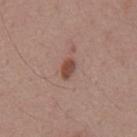{"biopsy_status": "not biopsied; imaged during a skin examination", "site": "mid back", "image": {"source": "total-body photography crop", "field_of_view_mm": 15}, "lighting": "white-light", "lesion_size": {"long_diameter_mm_approx": 2.5}, "automated_metrics": {"area_mm2_approx": 3.5, "eccentricity": 0.8, "border_irregularity_0_10": 1.5, "color_variation_0_10": 2.5, "peripheral_color_asymmetry": 1.0}, "patient": {"sex": "male", "age_approx": 55}}